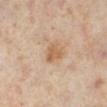  biopsy_status: not biopsied; imaged during a skin examination
  patient:
    sex: female
    age_approx: 50
  lighting: cross-polarized
  site: leg
  image:
    source: total-body photography crop
    field_of_view_mm: 15